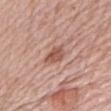Recorded during total-body skin imaging; not selected for excision or biopsy. This is a white-light tile. This image is a 15 mm lesion crop taken from a total-body photograph. From the right upper arm. Longest diameter approximately 3.5 mm. A female patient, about 65 years old.